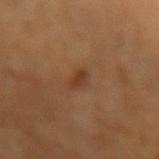{
  "biopsy_status": "not biopsied; imaged during a skin examination",
  "image": {
    "source": "total-body photography crop",
    "field_of_view_mm": 15
  },
  "site": "left forearm",
  "automated_metrics": {
    "eccentricity": 0.85,
    "shape_asymmetry": 0.3,
    "cielab_L": 36,
    "cielab_a": 21,
    "cielab_b": 32,
    "vs_skin_contrast_norm": 7.0,
    "color_variation_0_10": 0.5,
    "peripheral_color_asymmetry": 0.0
  },
  "lighting": "cross-polarized",
  "patient": {
    "age_approx": 70
  }
}